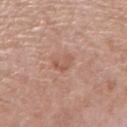Part of a total-body skin-imaging series; this lesion was reviewed on a skin check and was not flagged for biopsy. A roughly 15 mm field-of-view crop from a total-body skin photograph. A female subject, in their 70s. The recorded lesion diameter is about 3 mm. This is a white-light tile. The total-body-photography lesion software estimated a lesion area of about 3.5 mm² and a shape-asymmetry score of about 0.4 (0 = symmetric). The software also gave an average lesion color of about L≈56 a*≈22 b*≈29 (CIELAB), a lesion–skin lightness drop of about 7, and a normalized border contrast of about 5.5. It also reported a classifier nevus-likeness of about 0/100 and lesion-presence confidence of about 100/100. The lesion is on the right forearm.